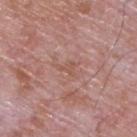image source: ~15 mm tile from a whole-body skin photo | size: ≈2.5 mm | subject: male, aged 63 to 67 | illumination: white-light | automated metrics: an average lesion color of about L≈54 a*≈22 b*≈26 (CIELAB), a lesion–skin lightness drop of about 6, and a lesion-to-skin contrast of about 5 (normalized; higher = more distinct); a border-irregularity index near 6.5/10, internal color variation of about 0 on a 0–10 scale, and a peripheral color-asymmetry measure near 0 | location: the upper back.